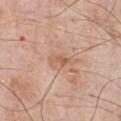| feature | finding |
|---|---|
| notes | imaged on a skin check; not biopsied |
| lesion size | ~2.5 mm (longest diameter) |
| patient | male, aged around 80 |
| image source | total-body-photography crop, ~15 mm field of view |
| tile lighting | white-light illumination |
| anatomic site | the chest |